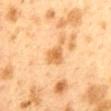The subject is a female approximately 40 years of age.
A 15 mm close-up tile from a total-body photography series done for melanoma screening.
The lesion is located on the mid back.